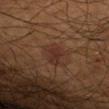Impression:
This lesion was catalogued during total-body skin photography and was not selected for biopsy.
Image and clinical context:
Cropped from a whole-body photographic skin survey; the tile spans about 15 mm. Captured under cross-polarized illumination. From the left forearm. A male subject, aged 63 to 67.